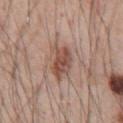Background:
On the chest. Longest diameter approximately 4.5 mm. The lesion-visualizer software estimated a border-irregularity rating of about 3/10 and a within-lesion color-variation index near 4.5/10. A lesion tile, about 15 mm wide, cut from a 3D total-body photograph. A male subject aged approximately 55. Imaged with white-light lighting.